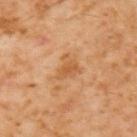Q: Was a biopsy performed?
A: imaged on a skin check; not biopsied
Q: Patient demographics?
A: male, aged around 60
Q: How was this image acquired?
A: 15 mm crop, total-body photography
Q: Automated lesion metrics?
A: an area of roughly 5.5 mm², an eccentricity of roughly 0.4, and a shape-asymmetry score of about 0.45 (0 = symmetric); a mean CIELAB color near L≈53 a*≈22 b*≈38, about 7 CIELAB-L* units darker than the surrounding skin, and a normalized border contrast of about 6
Q: What is the anatomic site?
A: the upper back
Q: Illumination type?
A: cross-polarized